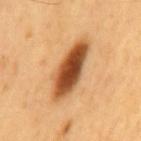<lesion>
  <biopsy_status>not biopsied; imaged during a skin examination</biopsy_status>
  <lighting>cross-polarized</lighting>
  <patient>
    <sex>male</sex>
    <age_approx>60</age_approx>
  </patient>
  <image>
    <source>total-body photography crop</source>
    <field_of_view_mm>15</field_of_view_mm>
  </image>
  <automated_metrics>
    <cielab_L>51</cielab_L>
    <cielab_a>25</cielab_a>
    <cielab_b>40</cielab_b>
    <vs_skin_darker_L>20.0</vs_skin_darker_L>
    <color_variation_0_10>8.5</color_variation_0_10>
    <nevus_likeness_0_100>90</nevus_likeness_0_100>
    <lesion_detection_confidence_0_100>100</lesion_detection_confidence_0_100>
  </automated_metrics>
  <site>mid back</site>
</lesion>